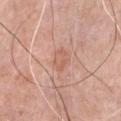| field | value |
|---|---|
| biopsy status | imaged on a skin check; not biopsied |
| body site | the chest |
| subject | male, aged 78 to 82 |
| image source | ~15 mm tile from a whole-body skin photo |
| size | ~2.5 mm (longest diameter) |
| tile lighting | white-light illumination |